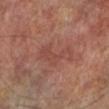Captured during whole-body skin photography for melanoma surveillance; the lesion was not biopsied.
This is a cross-polarized tile.
The subject is a female aged approximately 75.
Located on the leg.
A 15 mm crop from a total-body photograph taken for skin-cancer surveillance.